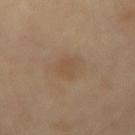Q: Was this lesion biopsied?
A: total-body-photography surveillance lesion; no biopsy
Q: Where on the body is the lesion?
A: the lower back
Q: Patient demographics?
A: female, aged 58 to 62
Q: What did automated image analysis measure?
A: an outline eccentricity of about 0.8 (0 = round, 1 = elongated) and a symmetry-axis asymmetry near 0.3; an average lesion color of about L≈50 a*≈15 b*≈31 (CIELAB) and about 5 CIELAB-L* units darker than the surrounding skin; a nevus-likeness score of about 5/100 and a lesion-detection confidence of about 100/100
Q: What is the imaging modality?
A: ~15 mm tile from a whole-body skin photo